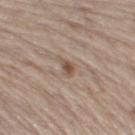Captured during whole-body skin photography for melanoma surveillance; the lesion was not biopsied. A male subject, aged approximately 70. The lesion is on the right thigh. Imaged with white-light lighting. The recorded lesion diameter is about 3 mm. The total-body-photography lesion software estimated border irregularity of about 4 on a 0–10 scale, a within-lesion color-variation index near 1.5/10, and a peripheral color-asymmetry measure near 0. The analysis additionally found a classifier nevus-likeness of about 0/100 and a lesion-detection confidence of about 100/100. A roughly 15 mm field-of-view crop from a total-body skin photograph.